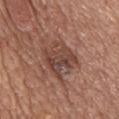The lesion was tiled from a total-body skin photograph and was not biopsied. Imaged with white-light lighting. On the chest. Longest diameter approximately 5 mm. A close-up tile cropped from a whole-body skin photograph, about 15 mm across. The total-body-photography lesion software estimated border irregularity of about 3 on a 0–10 scale, internal color variation of about 6 on a 0–10 scale, and a peripheral color-asymmetry measure near 2. The analysis additionally found a nevus-likeness score of about 30/100 and a detector confidence of about 100 out of 100 that the crop contains a lesion. The patient is a male aged around 60.